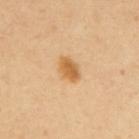Clinical impression: The lesion was photographed on a routine skin check and not biopsied; there is no pathology result.